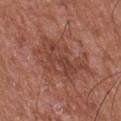Acquisition and patient details:
A 15 mm crop from a total-body photograph taken for skin-cancer surveillance. Imaged with white-light lighting. Longest diameter approximately 6.5 mm. A male patient, approximately 75 years of age. The lesion is located on the chest.